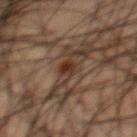follow-up: total-body-photography surveillance lesion; no biopsy | location: the mid back | illumination: cross-polarized | TBP lesion metrics: an outline eccentricity of about 0.9 (0 = round, 1 = elongated) and a symmetry-axis asymmetry near 0.35; a lesion color around L≈24 a*≈14 b*≈22 in CIELAB, a lesion–skin lightness drop of about 7, and a normalized lesion–skin contrast near 9; border irregularity of about 4 on a 0–10 scale, a within-lesion color-variation index near 1.5/10, and peripheral color asymmetry of about 0.5 | patient: male, in their 50s | acquisition: ~15 mm crop, total-body skin-cancer survey.